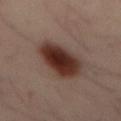Imaged during a routine full-body skin examination; the lesion was not biopsied and no histopathology is available.
This is a cross-polarized tile.
On the mid back.
A male subject approximately 40 years of age.
Cropped from a total-body skin-imaging series; the visible field is about 15 mm.
Longest diameter approximately 6 mm.
An algorithmic analysis of the crop reported a footprint of about 17 mm² and an eccentricity of roughly 0.8. It also reported a lesion color around L≈31 a*≈19 b*≈22 in CIELAB, a lesion–skin lightness drop of about 15, and a normalized border contrast of about 13.5.